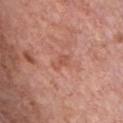Part of a total-body skin-imaging series; this lesion was reviewed on a skin check and was not flagged for biopsy. Automated image analysis of the tile measured a shape eccentricity near 0.85 and two-axis asymmetry of about 0.25. The analysis additionally found an average lesion color of about L≈54 a*≈27 b*≈30 (CIELAB), roughly 7 lightness units darker than nearby skin, and a lesion-to-skin contrast of about 5 (normalized; higher = more distinct). The software also gave a classifier nevus-likeness of about 0/100 and lesion-presence confidence of about 100/100. The tile uses white-light illumination. The lesion is located on the front of the torso. Measured at roughly 2.5 mm in maximum diameter. A 15 mm close-up extracted from a 3D total-body photography capture. A male subject aged 58–62.